Imaged with white-light lighting, the lesion is on the upper back, a 15 mm close-up tile from a total-body photography series done for melanoma screening, the lesion's longest dimension is about 8 mm, a male subject aged 48–52: 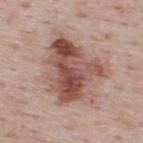Diagnosis:
The lesion was biopsied, and histopathology showed a dysplastic (Clark) nevus.